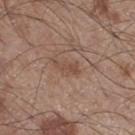Captured during whole-body skin photography for melanoma surveillance; the lesion was not biopsied. Approximately 3 mm at its widest. The tile uses white-light illumination. A male patient, aged 58 to 62. A 15 mm crop from a total-body photograph taken for skin-cancer surveillance. From the right thigh.